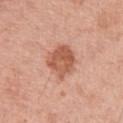<tbp_lesion>
  <biopsy_status>not biopsied; imaged during a skin examination</biopsy_status>
  <patient>
    <sex>female</sex>
    <age_approx>55</age_approx>
  </patient>
  <site>left upper arm</site>
  <lighting>white-light</lighting>
  <image>
    <source>total-body photography crop</source>
    <field_of_view_mm>15</field_of_view_mm>
  </image>
  <lesion_size>
    <long_diameter_mm_approx>4.0</long_diameter_mm_approx>
  </lesion_size>
</tbp_lesion>